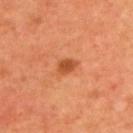Q: Was this lesion biopsied?
A: no biopsy performed (imaged during a skin exam)
Q: Patient demographics?
A: male, roughly 60 years of age
Q: Where on the body is the lesion?
A: the upper back
Q: How was this image acquired?
A: total-body-photography crop, ~15 mm field of view